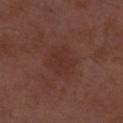- notes · imaged on a skin check; not biopsied
- acquisition · ~15 mm tile from a whole-body skin photo
- lesion size · ≈4 mm
- patient · male, in their 50s